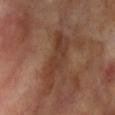Findings:
– notes · total-body-photography surveillance lesion; no biopsy
– imaging modality · 15 mm crop, total-body photography
– subject · female, aged 73–77
– diameter · ~6.5 mm (longest diameter)
– site · the arm
– automated metrics · a lesion color around L≈38 a*≈20 b*≈28 in CIELAB, about 7 CIELAB-L* units darker than the surrounding skin, and a normalized lesion–skin contrast near 6.5; a border-irregularity rating of about 5/10 and radial color variation of about 1; a classifier nevus-likeness of about 0/100 and a lesion-detection confidence of about 80/100
– lighting · cross-polarized illumination